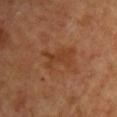Case summary:
– follow-up: no biopsy performed (imaged during a skin exam)
– lesion diameter: ≈4.5 mm
– location: the chest
– acquisition: ~15 mm tile from a whole-body skin photo
– patient: female, approximately 50 years of age
– lighting: cross-polarized illumination